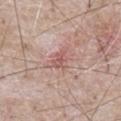| field | value |
|---|---|
| biopsy status | no biopsy performed (imaged during a skin exam) |
| automated metrics | a nevus-likeness score of about 0/100 and a lesion-detection confidence of about 100/100 |
| lighting | white-light illumination |
| patient | male, aged 63 to 67 |
| image source | ~15 mm crop, total-body skin-cancer survey |
| anatomic site | the abdomen |